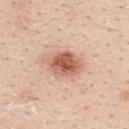{"biopsy_status": "not biopsied; imaged during a skin examination", "automated_metrics": {"eccentricity": 0.75, "shape_asymmetry": 0.15, "border_irregularity_0_10": 1.5, "color_variation_0_10": 6.0}, "lesion_size": {"long_diameter_mm_approx": 5.0}, "patient": {"sex": "female", "age_approx": 40}, "image": {"source": "total-body photography crop", "field_of_view_mm": 15}, "site": "upper back"}